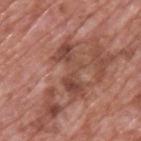  biopsy_status: not biopsied; imaged during a skin examination
  lighting: white-light
  image:
    source: total-body photography crop
    field_of_view_mm: 15
  site: upper back
  automated_metrics:
    cielab_L: 46
    cielab_a: 23
    cielab_b: 27
    vs_skin_darker_L: 10.0
    vs_skin_contrast_norm: 7.5
  patient:
    sex: male
    age_approx: 70